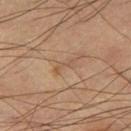Q: Is there a histopathology result?
A: catalogued during a skin exam; not biopsied
Q: What is the imaging modality?
A: ~15 mm crop, total-body skin-cancer survey
Q: Who is the patient?
A: male, approximately 60 years of age
Q: Lesion location?
A: the right thigh